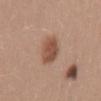notes: no biopsy performed (imaged during a skin exam) | diameter: ≈4 mm | image source: 15 mm crop, total-body photography | tile lighting: white-light | site: the mid back | subject: female, aged 23–27.